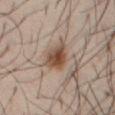Clinical impression:
Captured during whole-body skin photography for melanoma surveillance; the lesion was not biopsied.
Image and clinical context:
A 15 mm close-up extracted from a 3D total-body photography capture. The lesion is located on the abdomen. Captured under cross-polarized illumination. A male subject roughly 40 years of age.